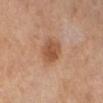<tbp_lesion>
  <biopsy_status>not biopsied; imaged during a skin examination</biopsy_status>
</tbp_lesion>